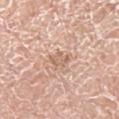* workup — no biopsy performed (imaged during a skin exam)
* image source — ~15 mm tile from a whole-body skin photo
* lesion diameter — ≈2.5 mm
* subject — male, about 75 years old
* location — the right lower leg
* image-analysis metrics — a shape eccentricity near 0.7; a border-irregularity rating of about 3/10, a within-lesion color-variation index near 1.5/10, and a peripheral color-asymmetry measure near 0.5; a nevus-likeness score of about 0/100 and a detector confidence of about 100 out of 100 that the crop contains a lesion
* tile lighting — white-light